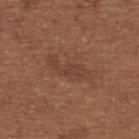Recorded during total-body skin imaging; not selected for excision or biopsy.
An algorithmic analysis of the crop reported a classifier nevus-likeness of about 0/100.
The patient is a female about 65 years old.
The lesion is located on the back.
A region of skin cropped from a whole-body photographic capture, roughly 15 mm wide.
Measured at roughly 5.5 mm in maximum diameter.